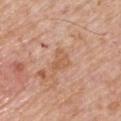follow-up: no biopsy performed (imaged during a skin exam)
patient: male, roughly 75 years of age
automated metrics: a mean CIELAB color near L≈58 a*≈22 b*≈34, roughly 7 lightness units darker than nearby skin, and a normalized lesion–skin contrast near 5.5
diameter: ~2.5 mm (longest diameter)
acquisition: ~15 mm crop, total-body skin-cancer survey
body site: the right upper arm